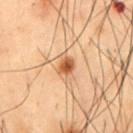Q: Was a biopsy performed?
A: catalogued during a skin exam; not biopsied